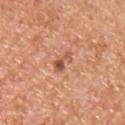workup: total-body-photography surveillance lesion; no biopsy
image: 15 mm crop, total-body photography
automated metrics: an area of roughly 3.5 mm², a shape eccentricity near 0.9, and a shape-asymmetry score of about 0.45 (0 = symmetric)
lesion diameter: ≈3.5 mm
lighting: white-light illumination
site: the front of the torso
patient: male, aged around 55